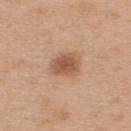follow-up: catalogued during a skin exam; not biopsied | automated metrics: a footprint of about 7 mm² and a symmetry-axis asymmetry near 0.2 | body site: the upper back | lighting: white-light | imaging modality: total-body-photography crop, ~15 mm field of view | subject: female, aged 38 to 42.